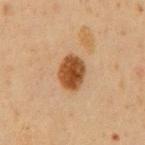This lesion was catalogued during total-body skin photography and was not selected for biopsy.
A roughly 15 mm field-of-view crop from a total-body skin photograph.
A male subject aged approximately 60.
The lesion is on the mid back.
Automated tile analysis of the lesion measured a lesion area of about 10 mm². And it measured an average lesion color of about L≈41 a*≈20 b*≈33 (CIELAB), about 14 CIELAB-L* units darker than the surrounding skin, and a normalized lesion–skin contrast near 12. And it measured a nevus-likeness score of about 100/100 and a lesion-detection confidence of about 100/100.
Imaged with cross-polarized lighting.